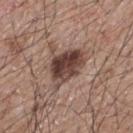The patient is a male aged around 65. The tile uses white-light illumination. A 15 mm close-up extracted from a 3D total-body photography capture. From the mid back. Approximately 5.5 mm at its widest. The total-body-photography lesion software estimated a lesion area of about 13 mm², a shape eccentricity near 0.8, and a shape-asymmetry score of about 0.3 (0 = symmetric).